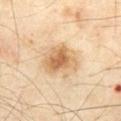biopsy_status: not biopsied; imaged during a skin examination
patient:
  sex: male
  age_approx: 70
lighting: cross-polarized
image:
  source: total-body photography crop
  field_of_view_mm: 15
lesion_size:
  long_diameter_mm_approx: 4.5
site: abdomen